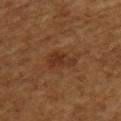biopsy_status: not biopsied; imaged during a skin examination
patient:
  sex: female
  age_approx: 55
automated_metrics:
  vs_skin_darker_L: 7.0
  vs_skin_contrast_norm: 7.0
  border_irregularity_0_10: 4.0
  color_variation_0_10: 3.0
  peripheral_color_asymmetry: 1.0
  nevus_likeness_0_100: 55
  lesion_detection_confidence_0_100: 100
lighting: cross-polarized
image:
  source: total-body photography crop
  field_of_view_mm: 15
lesion_size:
  long_diameter_mm_approx: 3.5
site: upper back